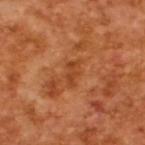notes — catalogued during a skin exam; not biopsied
subject — male, aged around 65
automated lesion analysis — an area of roughly 4 mm² and an eccentricity of roughly 0.85; a lesion color around L≈43 a*≈27 b*≈39 in CIELAB, a lesion–skin lightness drop of about 7, and a normalized lesion–skin contrast near 6; an automated nevus-likeness rating near 0 out of 100 and a lesion-detection confidence of about 100/100
lighting — cross-polarized illumination
size — ~3 mm (longest diameter)
image — 15 mm crop, total-body photography